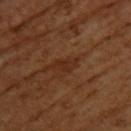Assessment: Imaged during a routine full-body skin examination; the lesion was not biopsied and no histopathology is available. Context: A female subject roughly 55 years of age. An algorithmic analysis of the crop reported a mean CIELAB color near L≈30 a*≈22 b*≈31, roughly 6 lightness units darker than nearby skin, and a normalized lesion–skin contrast near 6. The software also gave a border-irregularity rating of about 4/10, internal color variation of about 1 on a 0–10 scale, and a peripheral color-asymmetry measure near 0.5. The analysis additionally found a classifier nevus-likeness of about 0/100. The lesion is on the upper back. Measured at roughly 3 mm in maximum diameter. A region of skin cropped from a whole-body photographic capture, roughly 15 mm wide. The tile uses cross-polarized illumination.